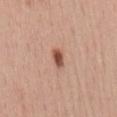Impression:
No biopsy was performed on this lesion — it was imaged during a full skin examination and was not determined to be concerning.
Clinical summary:
A 15 mm crop from a total-body photograph taken for skin-cancer surveillance. The subject is a male aged 38 to 42. The tile uses white-light illumination. The total-body-photography lesion software estimated lesion-presence confidence of about 100/100. Approximately 2.5 mm at its widest. Located on the mid back.